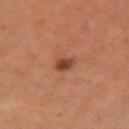Captured during whole-body skin photography for melanoma surveillance; the lesion was not biopsied. Longest diameter approximately 2 mm. On the left upper arm. A male subject, approximately 65 years of age. Cropped from a total-body skin-imaging series; the visible field is about 15 mm. Captured under cross-polarized illumination.